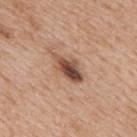• notes — catalogued during a skin exam; not biopsied
• image — 15 mm crop, total-body photography
• lighting — white-light illumination
• subject — male, about 65 years old
• body site — the upper back
• size — ~4.5 mm (longest diameter)